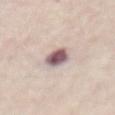acquisition — total-body-photography crop, ~15 mm field of view; patient — female, about 70 years old; body site — the abdomen; lesion size — ≈3.5 mm.